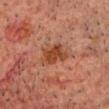biopsy status — total-body-photography surveillance lesion; no biopsy
patient — male, aged around 60
lesion size — about 3.5 mm
body site — the head or neck
image — 15 mm crop, total-body photography